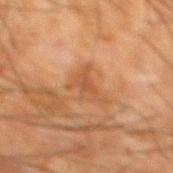Clinical summary: Automated tile analysis of the lesion measured a mean CIELAB color near L≈43 a*≈20 b*≈31, a lesion–skin lightness drop of about 7, and a normalized border contrast of about 5.5. It also reported a border-irregularity index near 7/10, a within-lesion color-variation index near 3/10, and radial color variation of about 1. The software also gave a classifier nevus-likeness of about 0/100 and lesion-presence confidence of about 100/100. A male patient, aged 63 to 67. From the mid back. Imaged with cross-polarized lighting. A lesion tile, about 15 mm wide, cut from a 3D total-body photograph. Measured at roughly 4 mm in maximum diameter.